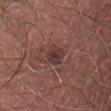* follow-up · catalogued during a skin exam; not biopsied
* image source · ~15 mm crop, total-body skin-cancer survey
* automated metrics · a footprint of about 5 mm² and a shape-asymmetry score of about 0.25 (0 = symmetric)
* site · the abdomen
* diameter · ≈2.5 mm
* subject · male, aged 58 to 62